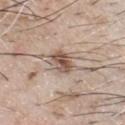Assessment: Recorded during total-body skin imaging; not selected for excision or biopsy. Image and clinical context: From the front of the torso. The patient is a male in their mid-60s. Cropped from a whole-body photographic skin survey; the tile spans about 15 mm. The lesion's longest dimension is about 3.5 mm. Automated tile analysis of the lesion measured a lesion area of about 7 mm², an eccentricity of roughly 0.65, and a shape-asymmetry score of about 0.25 (0 = symmetric). And it measured a lesion color around L≈54 a*≈15 b*≈25 in CIELAB, roughly 13 lightness units darker than nearby skin, and a normalized border contrast of about 9. The analysis additionally found border irregularity of about 2.5 on a 0–10 scale, a color-variation rating of about 5.5/10, and peripheral color asymmetry of about 1.5. It also reported a detector confidence of about 100 out of 100 that the crop contains a lesion. Captured under white-light illumination.